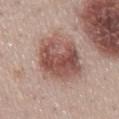Captured during whole-body skin photography for melanoma surveillance; the lesion was not biopsied.
A close-up tile cropped from a whole-body skin photograph, about 15 mm across.
From the back.
Approximately 6.5 mm at its widest.
A female patient, in their 50s.
The tile uses white-light illumination.